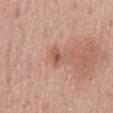workup — no biopsy performed (imaged during a skin exam)
body site — the mid back
patient — male, aged 63–67
TBP lesion metrics — border irregularity of about 3 on a 0–10 scale, internal color variation of about 3 on a 0–10 scale, and radial color variation of about 0.5
image — ~15 mm crop, total-body skin-cancer survey
lighting — white-light illumination
lesion diameter — ~2.5 mm (longest diameter)